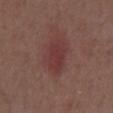This lesion was catalogued during total-body skin photography and was not selected for biopsy.
On the abdomen.
A male patient in their mid-50s.
A 15 mm crop from a total-body photograph taken for skin-cancer surveillance.